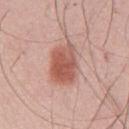{"biopsy_status": "not biopsied; imaged during a skin examination", "image": {"source": "total-body photography crop", "field_of_view_mm": 15}, "automated_metrics": {"area_mm2_approx": 14.0, "eccentricity": 0.6, "shape_asymmetry": 0.15, "cielab_L": 56, "cielab_a": 25, "cielab_b": 28, "border_irregularity_0_10": 1.5, "peripheral_color_asymmetry": 1.5}, "patient": {"sex": "male", "age_approx": 60}, "lighting": "white-light", "site": "front of the torso"}